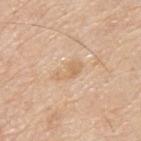The lesion was photographed on a routine skin check and not biopsied; there is no pathology result. This image is a 15 mm lesion crop taken from a total-body photograph. A male patient aged 78 to 82. The lesion is on the upper back.